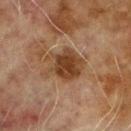Findings:
– notes — catalogued during a skin exam; not biopsied
– imaging modality — total-body-photography crop, ~15 mm field of view
– illumination — cross-polarized
– location — the right upper arm
– size — ~5 mm (longest diameter)
– subject — male, aged 68–72